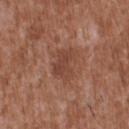Q: Who is the patient?
A: male, aged 43–47
Q: What is the imaging modality?
A: ~15 mm crop, total-body skin-cancer survey
Q: What is the anatomic site?
A: the back
Q: Illumination type?
A: white-light
Q: What did automated image analysis measure?
A: a footprint of about 9 mm², an eccentricity of roughly 0.7, and a shape-asymmetry score of about 0.25 (0 = symmetric); a lesion color around L≈44 a*≈23 b*≈28 in CIELAB, about 8 CIELAB-L* units darker than the surrounding skin, and a lesion-to-skin contrast of about 6 (normalized; higher = more distinct); a border-irregularity index near 3.5/10 and a within-lesion color-variation index near 2/10
Q: Lesion size?
A: ~4 mm (longest diameter)